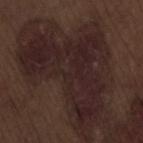Captured during whole-body skin photography for melanoma surveillance; the lesion was not biopsied.
A male subject, aged around 70.
The lesion is on the back.
An algorithmic analysis of the crop reported a classifier nevus-likeness of about 0/100 and lesion-presence confidence of about 60/100.
The recorded lesion diameter is about 12.5 mm.
This is a white-light tile.
A close-up tile cropped from a whole-body skin photograph, about 15 mm across.